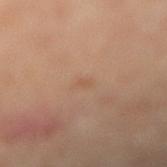Impression: The lesion was photographed on a routine skin check and not biopsied; there is no pathology result.